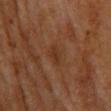Clinical impression: No biopsy was performed on this lesion — it was imaged during a full skin examination and was not determined to be concerning. Clinical summary: The lesion's longest dimension is about 2.5 mm. From the upper back. A female patient about 80 years old. A close-up tile cropped from a whole-body skin photograph, about 15 mm across. The tile uses cross-polarized illumination.